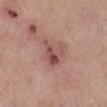Captured during whole-body skin photography for melanoma surveillance; the lesion was not biopsied. A 15 mm crop from a total-body photograph taken for skin-cancer surveillance. The total-body-photography lesion software estimated about 9 CIELAB-L* units darker than the surrounding skin and a normalized border contrast of about 7. From the right lower leg. Imaged with white-light lighting. About 4 mm across. A female patient roughly 55 years of age.